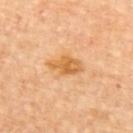{"biopsy_status": "not biopsied; imaged during a skin examination", "patient": {"sex": "female", "age_approx": 65}, "image": {"source": "total-body photography crop", "field_of_view_mm": 15}, "site": "upper back"}